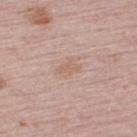Notes:
* follow-up · catalogued during a skin exam; not biopsied
* tile lighting · white-light
* image source · ~15 mm crop, total-body skin-cancer survey
* site · the upper back
* size · ~2.5 mm (longest diameter)
* patient · female, approximately 50 years of age